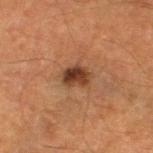Clinical impression: Captured during whole-body skin photography for melanoma surveillance; the lesion was not biopsied. Context: On the left lower leg. Automated image analysis of the tile measured a lesion area of about 5.5 mm², an eccentricity of roughly 0.7, and a shape-asymmetry score of about 0.3 (0 = symmetric). And it measured an average lesion color of about L≈32 a*≈19 b*≈27 (CIELAB) and roughly 12 lightness units darker than nearby skin. And it measured border irregularity of about 2.5 on a 0–10 scale, internal color variation of about 4.5 on a 0–10 scale, and peripheral color asymmetry of about 2. The software also gave a classifier nevus-likeness of about 90/100 and lesion-presence confidence of about 100/100. A male patient aged 63–67. This is a cross-polarized tile. A lesion tile, about 15 mm wide, cut from a 3D total-body photograph.